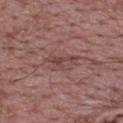The lesion is on the back. A male patient about 70 years old. A 15 mm close-up extracted from a 3D total-body photography capture. Imaged with white-light lighting. Automated tile analysis of the lesion measured a lesion color around L≈44 a*≈22 b*≈22 in CIELAB, about 7 CIELAB-L* units darker than the surrounding skin, and a normalized lesion–skin contrast near 6. The analysis additionally found a border-irregularity index near 6/10 and peripheral color asymmetry of about 1. The recorded lesion diameter is about 4 mm.